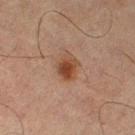  automated_metrics:
    area_mm2_approx: 6.0
    eccentricity: 0.65
    shape_asymmetry: 0.2
    cielab_L: 36
    cielab_a: 19
    cielab_b: 27
    vs_skin_darker_L: 9.0
    vs_skin_contrast_norm: 9.0
  patient:
    sex: male
    age_approx: 65
  image:
    source: total-body photography crop
    field_of_view_mm: 15
  lesion_size:
    long_diameter_mm_approx: 3.0
  site: left thigh
  lighting: cross-polarized